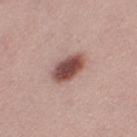The lesion was photographed on a routine skin check and not biopsied; there is no pathology result. On the leg. The lesion-visualizer software estimated a lesion-to-skin contrast of about 11.5 (normalized; higher = more distinct). It also reported an automated nevus-likeness rating near 100 out of 100 and lesion-presence confidence of about 100/100. Imaged with white-light lighting. A 15 mm close-up extracted from a 3D total-body photography capture. The subject is a female aged approximately 25.